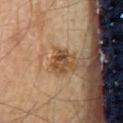  biopsy_status: not biopsied; imaged during a skin examination
  image:
    source: total-body photography crop
    field_of_view_mm: 15
  site: mid back
  patient:
    sex: male
    age_approx: 85+